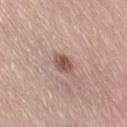  site: right thigh
  patient:
    sex: female
    age_approx: 65
  image:
    source: total-body photography crop
    field_of_view_mm: 15
  lighting: white-light